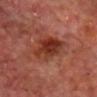The lesion was photographed on a routine skin check and not biopsied; there is no pathology result. The patient is a male approximately 65 years of age. Located on the head or neck. Cropped from a whole-body photographic skin survey; the tile spans about 15 mm.